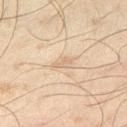Q: Was this lesion biopsied?
A: total-body-photography surveillance lesion; no biopsy
Q: What is the imaging modality?
A: total-body-photography crop, ~15 mm field of view
Q: What lighting was used for the tile?
A: cross-polarized
Q: What did automated image analysis measure?
A: two-axis asymmetry of about 0.5
Q: Patient demographics?
A: male, aged around 45
Q: How large is the lesion?
A: ≈2.5 mm
Q: Where on the body is the lesion?
A: the leg